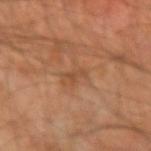Clinical impression:
The lesion was photographed on a routine skin check and not biopsied; there is no pathology result.
Acquisition and patient details:
This is a cross-polarized tile. About 2.5 mm across. A roughly 15 mm field-of-view crop from a total-body skin photograph. Located on the right forearm. The subject is a male in their mid- to late 50s.